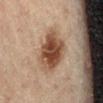Recorded during total-body skin imaging; not selected for excision or biopsy.
This image is a 15 mm lesion crop taken from a total-body photograph.
The patient is a male aged around 45.
Located on the lower back.
The lesion's longest dimension is about 5 mm.
This is a cross-polarized tile.